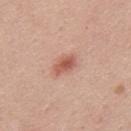Q: Automated lesion metrics?
A: a mean CIELAB color near L≈56 a*≈26 b*≈29, roughly 12 lightness units darker than nearby skin, and a normalized border contrast of about 8; a border-irregularity rating of about 2.5/10, a within-lesion color-variation index near 3.5/10, and radial color variation of about 1; a nevus-likeness score of about 90/100 and a lesion-detection confidence of about 100/100
Q: Illumination type?
A: white-light
Q: Patient demographics?
A: male, aged around 25
Q: How was this image acquired?
A: 15 mm crop, total-body photography
Q: How large is the lesion?
A: about 3 mm
Q: Where on the body is the lesion?
A: the mid back